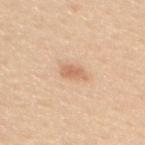A lesion tile, about 15 mm wide, cut from a 3D total-body photograph.
The patient is a female approximately 25 years of age.
On the back.
Captured under white-light illumination.